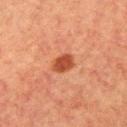Image and clinical context:
From the left upper arm. A female patient, in their mid-50s. Captured under cross-polarized illumination. This image is a 15 mm lesion crop taken from a total-body photograph. Longest diameter approximately 3 mm.